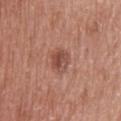Q: Is there a histopathology result?
A: no biopsy performed (imaged during a skin exam)
Q: What are the patient's age and sex?
A: male, aged around 60
Q: How large is the lesion?
A: ~3 mm (longest diameter)
Q: What is the imaging modality?
A: 15 mm crop, total-body photography
Q: Lesion location?
A: the upper back
Q: How was the tile lit?
A: white-light
Q: Automated lesion metrics?
A: border irregularity of about 1.5 on a 0–10 scale, a color-variation rating of about 5/10, and a peripheral color-asymmetry measure near 2